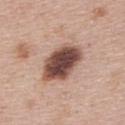notes: imaged on a skin check; not biopsied
body site: the upper back
lesion size: about 5.5 mm
TBP lesion metrics: a footprint of about 16 mm², an outline eccentricity of about 0.7 (0 = round, 1 = elongated), and a shape-asymmetry score of about 0.15 (0 = symmetric); a lesion-to-skin contrast of about 13.5 (normalized; higher = more distinct); a border-irregularity index near 2/10 and peripheral color asymmetry of about 1.5
image: 15 mm crop, total-body photography
subject: female, about 50 years old